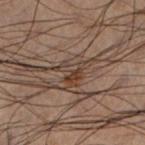The lesion was tiled from a total-body skin photograph and was not biopsied.
A male subject, in their 50s.
The lesion is located on the leg.
A 15 mm crop from a total-body photograph taken for skin-cancer surveillance.
The tile uses cross-polarized illumination.
The lesion-visualizer software estimated a footprint of about 4.5 mm² and a shape-asymmetry score of about 0.45 (0 = symmetric).